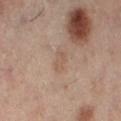The tile uses cross-polarized illumination. The lesion's longest dimension is about 2.5 mm. A 15 mm close-up extracted from a 3D total-body photography capture. The lesion is on the left leg. The patient is a female in their mid-50s.